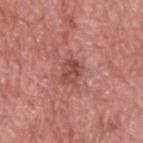Q: Was this lesion biopsied?
A: no biopsy performed (imaged during a skin exam)
Q: What is the anatomic site?
A: the back
Q: Automated lesion metrics?
A: an area of roughly 5.5 mm² and a shape eccentricity near 0.65
Q: Illumination type?
A: white-light illumination
Q: What is the lesion's diameter?
A: ~3 mm (longest diameter)
Q: Patient demographics?
A: male, roughly 60 years of age
Q: What kind of image is this?
A: total-body-photography crop, ~15 mm field of view